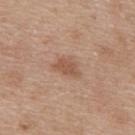Assessment:
Captured during whole-body skin photography for melanoma surveillance; the lesion was not biopsied.
Clinical summary:
The lesion is located on the back. A 15 mm close-up tile from a total-body photography series done for melanoma screening. This is a white-light tile. A female subject, roughly 40 years of age.